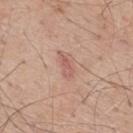Assessment: This lesion was catalogued during total-body skin photography and was not selected for biopsy. Context: A male subject, aged approximately 60. Located on the upper back. Longest diameter approximately 3 mm. Imaged with white-light lighting. A 15 mm close-up tile from a total-body photography series done for melanoma screening.